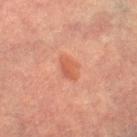<tbp_lesion>
<biopsy_status>not biopsied; imaged during a skin examination</biopsy_status>
<lesion_size>
  <long_diameter_mm_approx>3.0</long_diameter_mm_approx>
</lesion_size>
<image>
  <source>total-body photography crop</source>
  <field_of_view_mm>15</field_of_view_mm>
</image>
<patient>
  <sex>female</sex>
  <age_approx>60</age_approx>
</patient>
<site>right lower leg</site>
</tbp_lesion>